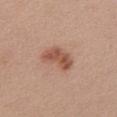<lesion>
  <biopsy_status>not biopsied; imaged during a skin examination</biopsy_status>
  <site>chest</site>
  <patient>
    <sex>female</sex>
    <age_approx>35</age_approx>
  </patient>
  <image>
    <source>total-body photography crop</source>
    <field_of_view_mm>15</field_of_view_mm>
  </image>
</lesion>